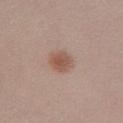patient:
  sex: female
  age_approx: 20
automated_metrics:
  eccentricity: 0.4
image:
  source: total-body photography crop
  field_of_view_mm: 15
site: left lower leg
lighting: white-light